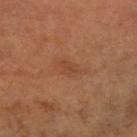acquisition = 15 mm crop, total-body photography; lesion diameter = ≈3 mm; patient = female, in their mid- to late 60s; site = the right forearm; lighting = cross-polarized illumination.